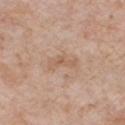Assessment: Recorded during total-body skin imaging; not selected for excision or biopsy. Image and clinical context: This is a white-light tile. The subject is a male aged around 60. A 15 mm crop from a total-body photograph taken for skin-cancer surveillance. The recorded lesion diameter is about 4 mm. On the chest.